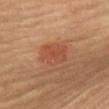{
  "biopsy_status": "not biopsied; imaged during a skin examination",
  "patient": {
    "sex": "female",
    "age_approx": 60
  },
  "lighting": "cross-polarized",
  "site": "chest",
  "automated_metrics": {
    "cielab_L": 45,
    "cielab_a": 25,
    "cielab_b": 31,
    "vs_skin_darker_L": 7.0,
    "vs_skin_contrast_norm": 5.0,
    "border_irregularity_0_10": 2.5,
    "color_variation_0_10": 4.0
  },
  "image": {
    "source": "total-body photography crop",
    "field_of_view_mm": 15
  }
}